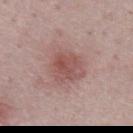Q: What did automated image analysis measure?
A: an area of roughly 8 mm², a shape eccentricity near 0.35, and two-axis asymmetry of about 0.25; a border-irregularity index near 3/10, a within-lesion color-variation index near 4/10, and a peripheral color-asymmetry measure near 1.5; a classifier nevus-likeness of about 40/100 and a detector confidence of about 100 out of 100 that the crop contains a lesion
Q: What kind of image is this?
A: ~15 mm crop, total-body skin-cancer survey
Q: What is the anatomic site?
A: the back
Q: Lesion size?
A: about 3.5 mm
Q: What are the patient's age and sex?
A: male, aged 48–52
Q: What lighting was used for the tile?
A: white-light illumination